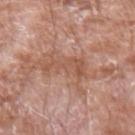This lesion was catalogued during total-body skin photography and was not selected for biopsy. Located on the arm. A lesion tile, about 15 mm wide, cut from a 3D total-body photograph. A male patient aged 58 to 62. The recorded lesion diameter is about 5 mm. Automated tile analysis of the lesion measured a mean CIELAB color near L≈54 a*≈22 b*≈29, a lesion–skin lightness drop of about 7, and a normalized border contrast of about 5.5. The analysis additionally found a within-lesion color-variation index near 3/10 and radial color variation of about 0.5. The software also gave an automated nevus-likeness rating near 0 out of 100 and a lesion-detection confidence of about 95/100.